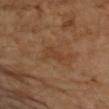biopsy status: no biopsy performed (imaged during a skin exam)
anatomic site: the left upper arm
subject: female, approximately 70 years of age
automated lesion analysis: a lesion area of about 5 mm² and an outline eccentricity of about 0.9 (0 = round, 1 = elongated)
imaging modality: ~15 mm tile from a whole-body skin photo